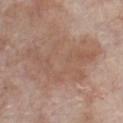biopsy status = total-body-photography surveillance lesion; no biopsy | body site = the front of the torso | image = ~15 mm tile from a whole-body skin photo | patient = male, aged around 65.